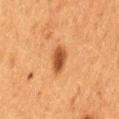{"biopsy_status": "not biopsied; imaged during a skin examination", "site": "lower back", "lesion_size": {"long_diameter_mm_approx": 3.5}, "automated_metrics": {"border_irregularity_0_10": 1.5, "peripheral_color_asymmetry": 1.0, "nevus_likeness_0_100": 95}, "image": {"source": "total-body photography crop", "field_of_view_mm": 15}, "lighting": "cross-polarized", "patient": {"sex": "female", "age_approx": 50}}